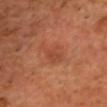Clinical impression: The lesion was tiled from a total-body skin photograph and was not biopsied. Background: The lesion is located on the head or neck. The tile uses cross-polarized illumination. Automated image analysis of the tile measured a lesion color around L≈46 a*≈28 b*≈33 in CIELAB and a lesion-to-skin contrast of about 4.5 (normalized; higher = more distinct). It also reported a lesion-detection confidence of about 100/100. About 3.5 mm across. A roughly 15 mm field-of-view crop from a total-body skin photograph. The subject is a male approximately 50 years of age.